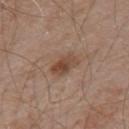Assessment: This lesion was catalogued during total-body skin photography and was not selected for biopsy. Context: Automated image analysis of the tile measured a lesion area of about 6 mm², an outline eccentricity of about 0.85 (0 = round, 1 = elongated), and two-axis asymmetry of about 0.2. The software also gave a border-irregularity index near 2.5/10, a within-lesion color-variation index near 5/10, and peripheral color asymmetry of about 1.5. The lesion's longest dimension is about 3.5 mm. Imaged with white-light lighting. A male patient, aged around 55. The lesion is located on the mid back. A region of skin cropped from a whole-body photographic capture, roughly 15 mm wide.